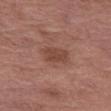Notes:
- notes: imaged on a skin check; not biopsied
- illumination: white-light illumination
- lesion size: ≈3 mm
- body site: the left thigh
- subject: male, about 80 years old
- acquisition: ~15 mm crop, total-body skin-cancer survey